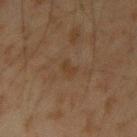The lesion was photographed on a routine skin check and not biopsied; there is no pathology result. A male patient aged 43 to 47. From the left forearm. Cropped from a whole-body photographic skin survey; the tile spans about 15 mm.